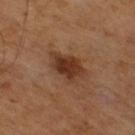Q: Was this lesion biopsied?
A: imaged on a skin check; not biopsied
Q: What kind of image is this?
A: ~15 mm crop, total-body skin-cancer survey
Q: How large is the lesion?
A: ~4.5 mm (longest diameter)
Q: What are the patient's age and sex?
A: male, in their mid- to late 60s
Q: Automated lesion metrics?
A: an outline eccentricity of about 0.7 (0 = round, 1 = elongated) and a symmetry-axis asymmetry near 0.25; an average lesion color of about L≈34 a*≈21 b*≈29 (CIELAB); lesion-presence confidence of about 100/100
Q: How was the tile lit?
A: cross-polarized illumination